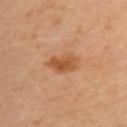| key | value |
|---|---|
| follow-up | imaged on a skin check; not biopsied |
| anatomic site | the upper back |
| size | about 4 mm |
| image-analysis metrics | an area of roughly 7.5 mm² and an eccentricity of roughly 0.85; a lesion color around L≈55 a*≈25 b*≈38 in CIELAB and a normalized border contrast of about 7.5; a border-irregularity rating of about 2/10 and peripheral color asymmetry of about 1.5; a classifier nevus-likeness of about 75/100 and a detector confidence of about 100 out of 100 that the crop contains a lesion |
| image | ~15 mm crop, total-body skin-cancer survey |
| lighting | cross-polarized illumination |
| subject | female, aged 38 to 42 |